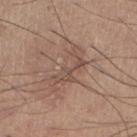Clinical impression:
Recorded during total-body skin imaging; not selected for excision or biopsy.
Background:
A region of skin cropped from a whole-body photographic capture, roughly 15 mm wide. Automated tile analysis of the lesion measured an area of roughly 10 mm², an outline eccentricity of about 0.9 (0 = round, 1 = elongated), and a symmetry-axis asymmetry near 0.5. The analysis additionally found border irregularity of about 7 on a 0–10 scale, internal color variation of about 3.5 on a 0–10 scale, and radial color variation of about 1.5. And it measured a lesion-detection confidence of about 80/100. The patient is a male roughly 55 years of age. The tile uses white-light illumination. From the right lower leg. The lesion's longest dimension is about 5.5 mm.